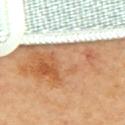follow-up=total-body-photography surveillance lesion; no biopsy | location=the back | TBP lesion metrics=an average lesion color of about L≈64 a*≈20 b*≈38 (CIELAB) and a lesion–skin lightness drop of about 16; border irregularity of about 5 on a 0–10 scale and internal color variation of about 8.5 on a 0–10 scale; an automated nevus-likeness rating near 100 out of 100 and lesion-presence confidence of about 100/100 | lesion diameter=about 13.5 mm | lighting=cross-polarized | image=15 mm crop, total-body photography | patient=female.